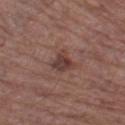Case summary:
– notes — catalogued during a skin exam; not biopsied
– subject — male, in their mid-60s
– body site — the left thigh
– size — ≈3 mm
– automated lesion analysis — a footprint of about 5 mm², a shape eccentricity near 0.65, and a shape-asymmetry score of about 0.35 (0 = symmetric); a border-irregularity index near 4/10, a within-lesion color-variation index near 3/10, and a peripheral color-asymmetry measure near 1
– imaging modality — total-body-photography crop, ~15 mm field of view
– tile lighting — white-light illumination